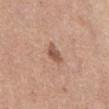notes = total-body-photography surveillance lesion; no biopsy | lesion size = about 2.5 mm | imaging modality = total-body-photography crop, ~15 mm field of view | subject = female, aged 53 to 57 | anatomic site = the abdomen | automated metrics = a footprint of about 4 mm² and a symmetry-axis asymmetry near 0.3; a lesion–skin lightness drop of about 11 | illumination = white-light illumination.